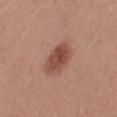| field | value |
|---|---|
| follow-up | catalogued during a skin exam; not biopsied |
| illumination | white-light |
| image source | total-body-photography crop, ~15 mm field of view |
| subject | female, aged approximately 55 |
| body site | the leg |